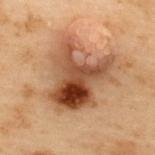Findings:
* notes: imaged on a skin check; not biopsied
* image: total-body-photography crop, ~15 mm field of view
* patient: male, in their mid-50s
* body site: the upper back